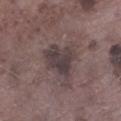Findings:
• workup — no biopsy performed (imaged during a skin exam)
• subject — male, approximately 75 years of age
• acquisition — total-body-photography crop, ~15 mm field of view
• anatomic site — the left lower leg